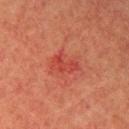The lesion was photographed on a routine skin check and not biopsied; there is no pathology result. Imaged with cross-polarized lighting. Automated tile analysis of the lesion measured a lesion color around L≈37 a*≈32 b*≈28 in CIELAB, about 6 CIELAB-L* units darker than the surrounding skin, and a normalized border contrast of about 5.5. It also reported border irregularity of about 5 on a 0–10 scale, a color-variation rating of about 3/10, and peripheral color asymmetry of about 1. And it measured a classifier nevus-likeness of about 0/100 and lesion-presence confidence of about 100/100. The lesion is located on the right upper arm. The lesion's longest dimension is about 3.5 mm. A female subject, aged 53–57. A 15 mm crop from a total-body photograph taken for skin-cancer surveillance.